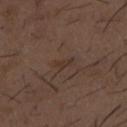Notes:
- biopsy status: catalogued during a skin exam; not biopsied
- location: the mid back
- patient: male, aged 48–52
- lighting: white-light
- image source: ~15 mm crop, total-body skin-cancer survey